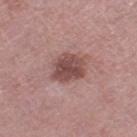Imaged during a routine full-body skin examination; the lesion was not biopsied and no histopathology is available. The lesion is on the leg. A 15 mm crop from a total-body photograph taken for skin-cancer surveillance. Captured under white-light illumination. The lesion-visualizer software estimated an area of roughly 11 mm², an outline eccentricity of about 0.65 (0 = round, 1 = elongated), and two-axis asymmetry of about 0.2. And it measured an average lesion color of about L≈48 a*≈22 b*≈21 (CIELAB), about 12 CIELAB-L* units darker than the surrounding skin, and a normalized lesion–skin contrast near 9. Measured at roughly 4.5 mm in maximum diameter. A female patient, in their 70s.